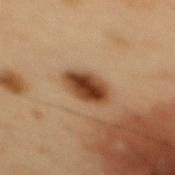Impression: Captured during whole-body skin photography for melanoma surveillance; the lesion was not biopsied. Image and clinical context: The lesion's longest dimension is about 4 mm. The subject is a male in their mid-50s. A region of skin cropped from a whole-body photographic capture, roughly 15 mm wide. The tile uses cross-polarized illumination. The lesion is located on the mid back. Automated image analysis of the tile measured an area of roughly 9 mm², an eccentricity of roughly 0.75, and a symmetry-axis asymmetry near 0.15. The analysis additionally found roughly 14 lightness units darker than nearby skin and a normalized lesion–skin contrast near 12.5. And it measured a border-irregularity rating of about 1.5/10, a color-variation rating of about 5.5/10, and peripheral color asymmetry of about 2.